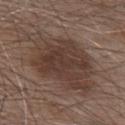The lesion was tiled from a total-body skin photograph and was not biopsied. A male subject approximately 80 years of age. The total-body-photography lesion software estimated a footprint of about 42 mm². And it measured a nevus-likeness score of about 30/100 and a lesion-detection confidence of about 100/100. On the chest. Cropped from a total-body skin-imaging series; the visible field is about 15 mm.